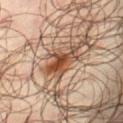No biopsy was performed on this lesion — it was imaged during a full skin examination and was not determined to be concerning. The lesion-visualizer software estimated a lesion area of about 6.5 mm², a shape eccentricity near 0.8, and two-axis asymmetry of about 0.5. It also reported a lesion color around L≈45 a*≈22 b*≈31 in CIELAB, roughly 13 lightness units darker than nearby skin, and a normalized lesion–skin contrast near 10.5. It also reported lesion-presence confidence of about 100/100. The lesion is located on the left thigh. A male patient in their mid- to late 60s. Cropped from a total-body skin-imaging series; the visible field is about 15 mm. The recorded lesion diameter is about 4 mm. Captured under cross-polarized illumination.